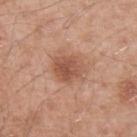workup — total-body-photography surveillance lesion; no biopsy | subject — male, aged around 55 | site — the arm | image source — total-body-photography crop, ~15 mm field of view | lighting — white-light.